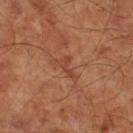Recorded during total-body skin imaging; not selected for excision or biopsy. A male patient aged 58 to 62. Cropped from a whole-body photographic skin survey; the tile spans about 15 mm. The lesion is on the right lower leg. Automated tile analysis of the lesion measured a lesion color around L≈42 a*≈26 b*≈31 in CIELAB, a lesion–skin lightness drop of about 7, and a normalized border contrast of about 5.5. And it measured a nevus-likeness score of about 0/100 and a lesion-detection confidence of about 95/100. The lesion's longest dimension is about 2.5 mm. This is a cross-polarized tile.